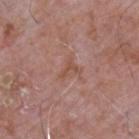Clinical impression: The lesion was photographed on a routine skin check and not biopsied; there is no pathology result. Background: The total-body-photography lesion software estimated a border-irregularity index near 7/10 and radial color variation of about 0.5. It also reported a classifier nevus-likeness of about 0/100 and a lesion-detection confidence of about 100/100. The patient is a male aged 63–67. A close-up tile cropped from a whole-body skin photograph, about 15 mm across. The lesion is located on the upper back.